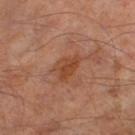follow-up: imaged on a skin check; not biopsied
patient: male, aged approximately 65
body site: the right lower leg
acquisition: 15 mm crop, total-body photography